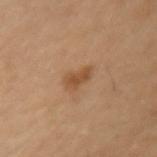workup: no biopsy performed (imaged during a skin exam)
image: 15 mm crop, total-body photography
lesion size: ~3 mm (longest diameter)
patient: female, approximately 50 years of age
site: the left upper arm
tile lighting: cross-polarized
automated lesion analysis: a border-irregularity rating of about 3/10 and internal color variation of about 1.5 on a 0–10 scale; an automated nevus-likeness rating near 65 out of 100